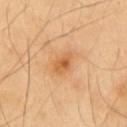{"biopsy_status": "not biopsied; imaged during a skin examination", "image": {"source": "total-body photography crop", "field_of_view_mm": 15}, "lighting": "cross-polarized", "lesion_size": {"long_diameter_mm_approx": 3.0}, "site": "front of the torso"}